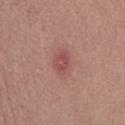Part of a total-body skin-imaging series; this lesion was reviewed on a skin check and was not flagged for biopsy. The recorded lesion diameter is about 3 mm. On the chest. A male patient, aged approximately 30. A lesion tile, about 15 mm wide, cut from a 3D total-body photograph.